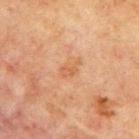The lesion is on the back.
A male patient roughly 70 years of age.
A 15 mm crop from a total-body photograph taken for skin-cancer surveillance.